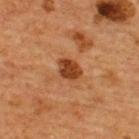| key | value |
|---|---|
| patient | male, in their mid-60s |
| lesion size | about 3 mm |
| site | the upper back |
| image source | ~15 mm tile from a whole-body skin photo |
| lighting | cross-polarized illumination |
| image-analysis metrics | an area of roughly 5.5 mm², an outline eccentricity of about 0.65 (0 = round, 1 = elongated), and a symmetry-axis asymmetry near 0.2; an average lesion color of about L≈36 a*≈24 b*≈34 (CIELAB), about 12 CIELAB-L* units darker than the surrounding skin, and a lesion-to-skin contrast of about 10 (normalized; higher = more distinct); a border-irregularity index near 2/10, internal color variation of about 2.5 on a 0–10 scale, and peripheral color asymmetry of about 1; a classifier nevus-likeness of about 90/100 and lesion-presence confidence of about 100/100 |